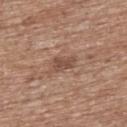workup — total-body-photography surveillance lesion; no biopsy
subject — male, approximately 70 years of age
image — total-body-photography crop, ~15 mm field of view
anatomic site — the back
tile lighting — white-light
lesion diameter — ~3 mm (longest diameter)